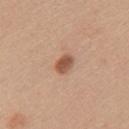  biopsy_status: not biopsied; imaged during a skin examination
  patient:
    sex: female
    age_approx: 35
  image:
    source: total-body photography crop
    field_of_view_mm: 15
  lesion_size:
    long_diameter_mm_approx: 2.5
  site: left upper arm
  lighting: white-light